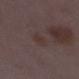Impression: No biopsy was performed on this lesion — it was imaged during a full skin examination and was not determined to be concerning. Context: A female patient aged 28 to 32. Measured at roughly 2.5 mm in maximum diameter. This image is a 15 mm lesion crop taken from a total-body photograph. An algorithmic analysis of the crop reported a lesion area of about 4.5 mm², an outline eccentricity of about 0.35 (0 = round, 1 = elongated), and two-axis asymmetry of about 0.15. It also reported a border-irregularity rating of about 1.5/10, internal color variation of about 1.5 on a 0–10 scale, and a peripheral color-asymmetry measure near 0.5. The lesion is on the left lower leg. Imaged with white-light lighting.